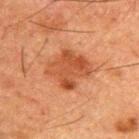Imaged during a routine full-body skin examination; the lesion was not biopsied and no histopathology is available.